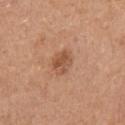| key | value |
|---|---|
| follow-up | total-body-photography surveillance lesion; no biopsy |
| imaging modality | ~15 mm tile from a whole-body skin photo |
| lighting | white-light |
| patient | male, aged 73–77 |
| anatomic site | the arm |
| size | ~3 mm (longest diameter) |